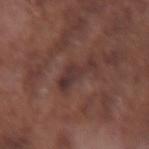biopsy_status: not biopsied; imaged during a skin examination
lighting: white-light
automated_metrics:
  cielab_L: 33
  cielab_a: 18
  cielab_b: 19
  vs_skin_darker_L: 7.0
  vs_skin_contrast_norm: 7.5
  nevus_likeness_0_100: 0
  lesion_detection_confidence_0_100: 70
image:
  source: total-body photography crop
  field_of_view_mm: 15
patient:
  sex: male
  age_approx: 75
lesion_size:
  long_diameter_mm_approx: 4.5
site: arm